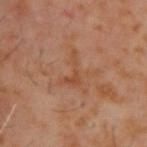{"biopsy_status": "not biopsied; imaged during a skin examination", "patient": {"sex": "male", "age_approx": 60}, "site": "upper back", "lighting": "cross-polarized", "lesion_size": {"long_diameter_mm_approx": 4.0}, "image": {"source": "total-body photography crop", "field_of_view_mm": 15}}